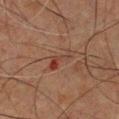| feature | finding |
|---|---|
| notes | total-body-photography surveillance lesion; no biopsy |
| patient | male, in their 60s |
| automated lesion analysis | a footprint of about 4.5 mm² and an outline eccentricity of about 0.9 (0 = round, 1 = elongated); a lesion color around L≈33 a*≈20 b*≈24 in CIELAB, about 6 CIELAB-L* units darker than the surrounding skin, and a lesion-to-skin contrast of about 6.5 (normalized; higher = more distinct); a border-irregularity index near 5.5/10, a within-lesion color-variation index near 4.5/10, and radial color variation of about 1; a lesion-detection confidence of about 100/100 |
| lighting | cross-polarized |
| anatomic site | the front of the torso |
| size | ≈3.5 mm |
| imaging modality | ~15 mm tile from a whole-body skin photo |